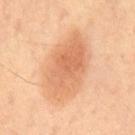Captured during whole-body skin photography for melanoma surveillance; the lesion was not biopsied.
A male patient, in their mid-50s.
The lesion-visualizer software estimated a mean CIELAB color near L≈67 a*≈24 b*≈38, roughly 11 lightness units darker than nearby skin, and a normalized lesion–skin contrast near 6.5.
The tile uses cross-polarized illumination.
Located on the mid back.
A lesion tile, about 15 mm wide, cut from a 3D total-body photograph.